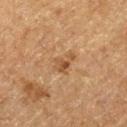* notes: imaged on a skin check; not biopsied
* acquisition: total-body-photography crop, ~15 mm field of view
* location: the leg
* patient: male, aged 73 to 77